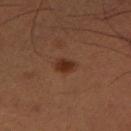Recorded during total-body skin imaging; not selected for excision or biopsy. Imaged with cross-polarized lighting. Automated tile analysis of the lesion measured a shape eccentricity near 0.75 and a shape-asymmetry score of about 0.25 (0 = symmetric). It also reported a lesion color around L≈31 a*≈21 b*≈29 in CIELAB. It also reported a border-irregularity index near 2/10, internal color variation of about 2.5 on a 0–10 scale, and radial color variation of about 0.5. The recorded lesion diameter is about 2.5 mm. A male patient about 50 years old. Cropped from a total-body skin-imaging series; the visible field is about 15 mm. From the left thigh.